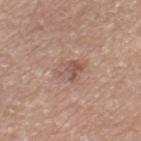Imaged during a routine full-body skin examination; the lesion was not biopsied and no histopathology is available.
A female patient in their mid- to late 70s.
Located on the left thigh.
Cropped from a whole-body photographic skin survey; the tile spans about 15 mm.
Automated tile analysis of the lesion measured a classifier nevus-likeness of about 0/100 and lesion-presence confidence of about 100/100.
The tile uses white-light illumination.
Approximately 3.5 mm at its widest.